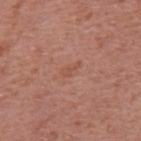Findings:
- workup · total-body-photography surveillance lesion; no biopsy
- image-analysis metrics · a footprint of about 2.5 mm², a shape eccentricity near 0.85, and two-axis asymmetry of about 0.35; a border-irregularity rating of about 3.5/10, a color-variation rating of about 0.5/10, and radial color variation of about 0
- image · ~15 mm crop, total-body skin-cancer survey
- site · the mid back
- patient · male, aged 68 to 72
- illumination · white-light illumination
- lesion size · ≈2.5 mm